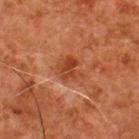Part of a total-body skin-imaging series; this lesion was reviewed on a skin check and was not flagged for biopsy.
The lesion is located on the upper back.
This image is a 15 mm lesion crop taken from a total-body photograph.
A male subject, in their 80s.